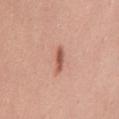Case summary:
- biopsy status: catalogued during a skin exam; not biopsied
- tile lighting: white-light illumination
- patient: female, roughly 35 years of age
- anatomic site: the abdomen
- size: about 3 mm
- acquisition: ~15 mm crop, total-body skin-cancer survey
- image-analysis metrics: a lesion area of about 3 mm² and a shape-asymmetry score of about 0.2 (0 = symmetric); a mean CIELAB color near L≈56 a*≈26 b*≈30 and a normalized border contrast of about 8.5